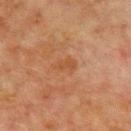The lesion was photographed on a routine skin check and not biopsied; there is no pathology result.
A male subject in their 70s.
Located on the chest.
Imaged with cross-polarized lighting.
The total-body-photography lesion software estimated a mean CIELAB color near L≈42 a*≈21 b*≈32, a lesion–skin lightness drop of about 5, and a normalized border contrast of about 5.
Measured at roughly 3 mm in maximum diameter.
A region of skin cropped from a whole-body photographic capture, roughly 15 mm wide.